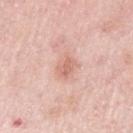  biopsy_status: not biopsied; imaged during a skin examination
  image:
    source: total-body photography crop
    field_of_view_mm: 15
  site: left upper arm
  patient:
    sex: female
    age_approx: 65
  lighting: white-light